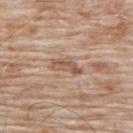  biopsy_status: not biopsied; imaged during a skin examination
  patient:
    sex: male
    age_approx: 80
  automated_metrics:
    area_mm2_approx: 5.0
    eccentricity: 0.9
    shape_asymmetry: 0.3
    border_irregularity_0_10: 3.5
    color_variation_0_10: 3.0
    peripheral_color_asymmetry: 1.0
    nevus_likeness_0_100: 0
  image:
    source: total-body photography crop
    field_of_view_mm: 15
  lesion_size:
    long_diameter_mm_approx: 4.0
  lighting: white-light
  site: upper back